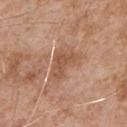Part of a total-body skin-imaging series; this lesion was reviewed on a skin check and was not flagged for biopsy. Automated tile analysis of the lesion measured a footprint of about 7.5 mm² and a shape eccentricity near 0.8. The analysis additionally found a lesion color around L≈53 a*≈21 b*≈32 in CIELAB, about 9 CIELAB-L* units darker than the surrounding skin, and a normalized border contrast of about 6.5. The software also gave a border-irregularity index near 4.5/10 and a within-lesion color-variation index near 2/10. From the chest. Longest diameter approximately 4 mm. Cropped from a total-body skin-imaging series; the visible field is about 15 mm. The patient is a male about 65 years old.